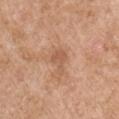Assessment: The lesion was photographed on a routine skin check and not biopsied; there is no pathology result. Context: Located on the right upper arm. The recorded lesion diameter is about 3 mm. A male patient approximately 55 years of age. This image is a 15 mm lesion crop taken from a total-body photograph. This is a white-light tile.